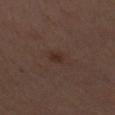The lesion was tiled from a total-body skin photograph and was not biopsied. The subject is a female about 50 years old. Captured under cross-polarized illumination. A roughly 15 mm field-of-view crop from a total-body skin photograph. The lesion is on the right upper arm.